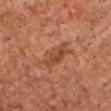The lesion was photographed on a routine skin check and not biopsied; there is no pathology result. Measured at roughly 4.5 mm in maximum diameter. From the head or neck. A male subject, aged 58 to 62. A close-up tile cropped from a whole-body skin photograph, about 15 mm across.